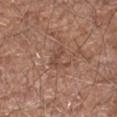Part of a total-body skin-imaging series; this lesion was reviewed on a skin check and was not flagged for biopsy.
A region of skin cropped from a whole-body photographic capture, roughly 15 mm wide.
Captured under white-light illumination.
A male patient, aged 58 to 62.
About 3 mm across.
The lesion is on the right forearm.